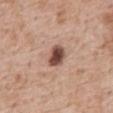Clinical impression: The lesion was photographed on a routine skin check and not biopsied; there is no pathology result. Acquisition and patient details: Located on the abdomen. This image is a 15 mm lesion crop taken from a total-body photograph. The lesion's longest dimension is about 3 mm. A male patient, about 60 years old. The total-body-photography lesion software estimated an outline eccentricity of about 0.65 (0 = round, 1 = elongated) and two-axis asymmetry of about 0.2. The analysis additionally found a mean CIELAB color near L≈48 a*≈21 b*≈26 and a normalized lesion–skin contrast near 11.5. And it measured a classifier nevus-likeness of about 85/100 and lesion-presence confidence of about 100/100.